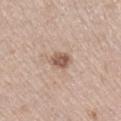lesion size=about 2.5 mm; patient=female, aged around 70; anatomic site=the right lower leg; tile lighting=white-light illumination; imaging modality=~15 mm tile from a whole-body skin photo.